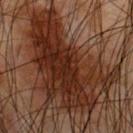No biopsy was performed on this lesion — it was imaged during a full skin examination and was not determined to be concerning.
A 15 mm close-up tile from a total-body photography series done for melanoma screening.
A male subject, in their mid- to late 60s.
The lesion is on the chest.
This is a cross-polarized tile.